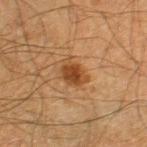Imaged during a routine full-body skin examination; the lesion was not biopsied and no histopathology is available. The lesion is located on the left thigh. This image is a 15 mm lesion crop taken from a total-body photograph. A male patient aged 63–67. Captured under cross-polarized illumination. An algorithmic analysis of the crop reported two-axis asymmetry of about 0.25. The software also gave a lesion–skin lightness drop of about 10. The software also gave a border-irregularity rating of about 3/10, a color-variation rating of about 3/10, and radial color variation of about 1. About 3.5 mm across.